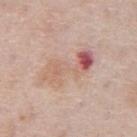Clinical impression:
Part of a total-body skin-imaging series; this lesion was reviewed on a skin check and was not flagged for biopsy.
Context:
Imaged with white-light lighting. A male subject, roughly 75 years of age. The lesion is on the abdomen. About 7 mm across. A 15 mm crop from a total-body photograph taken for skin-cancer surveillance.